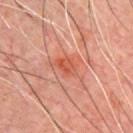follow-up: no biopsy performed (imaged during a skin exam)
site: the front of the torso
diameter: about 2.5 mm
patient: male, about 45 years old
image: 15 mm crop, total-body photography
TBP lesion metrics: a border-irregularity rating of about 2/10, a within-lesion color-variation index near 3.5/10, and peripheral color asymmetry of about 1; a nevus-likeness score of about 35/100
tile lighting: cross-polarized